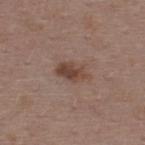lesion size = about 4 mm
automated lesion analysis = a mean CIELAB color near L≈44 a*≈18 b*≈24 and a normalized border contrast of about 8; a classifier nevus-likeness of about 60/100 and a detector confidence of about 100 out of 100 that the crop contains a lesion
lighting = white-light illumination
image source = total-body-photography crop, ~15 mm field of view
patient = female, approximately 45 years of age
site = the upper back